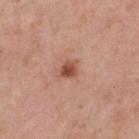Part of a total-body skin-imaging series; this lesion was reviewed on a skin check and was not flagged for biopsy.
Longest diameter approximately 2.5 mm.
A close-up tile cropped from a whole-body skin photograph, about 15 mm across.
A male patient, aged 53 to 57.
Imaged with white-light lighting.
The lesion is located on the back.